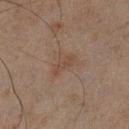No biopsy was performed on this lesion — it was imaged during a full skin examination and was not determined to be concerning.
An algorithmic analysis of the crop reported a color-variation rating of about 1/10 and a peripheral color-asymmetry measure near 0.5.
A 15 mm crop from a total-body photograph taken for skin-cancer surveillance.
The subject is a male in their mid-40s.
Captured under cross-polarized illumination.
On the left lower leg.